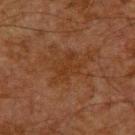biopsy status = catalogued during a skin exam; not biopsied | subject = male, in their 60s | image source = ~15 mm tile from a whole-body skin photo | anatomic site = the upper back.